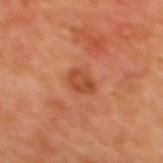Assessment:
This lesion was catalogued during total-body skin photography and was not selected for biopsy.
Clinical summary:
A region of skin cropped from a whole-body photographic capture, roughly 15 mm wide. The subject is a female aged approximately 40. This is a cross-polarized tile. The lesion is located on the upper back. Automated image analysis of the tile measured border irregularity of about 2 on a 0–10 scale and a within-lesion color-variation index near 3.5/10.